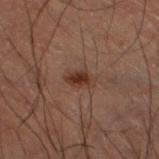notes: no biopsy performed (imaged during a skin exam).